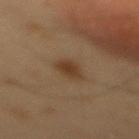The lesion was photographed on a routine skin check and not biopsied; there is no pathology result.
A close-up tile cropped from a whole-body skin photograph, about 15 mm across.
The lesion is located on the mid back.
An algorithmic analysis of the crop reported an outline eccentricity of about 0.8 (0 = round, 1 = elongated) and a symmetry-axis asymmetry near 0.2. The software also gave a lesion color around L≈30 a*≈14 b*≈25 in CIELAB, roughly 7 lightness units darker than nearby skin, and a lesion-to-skin contrast of about 8 (normalized; higher = more distinct).
Longest diameter approximately 3 mm.
Captured under cross-polarized illumination.
A male patient, aged around 55.